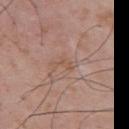<record>
<biopsy_status>not biopsied; imaged during a skin examination</biopsy_status>
<site>upper back</site>
<image>
  <source>total-body photography crop</source>
  <field_of_view_mm>15</field_of_view_mm>
</image>
<automated_metrics>
  <border_irregularity_0_10>5.5</border_irregularity_0_10>
  <color_variation_0_10>0.0</color_variation_0_10>
  <peripheral_color_asymmetry>0.0</peripheral_color_asymmetry>
  <nevus_likeness_0_100>0</nevus_likeness_0_100>
  <lesion_detection_confidence_0_100>95</lesion_detection_confidence_0_100>
</automated_metrics>
<lighting>white-light</lighting>
<patient>
  <sex>male</sex>
  <age_approx>55</age_approx>
</patient>
</record>